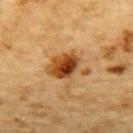biopsy_status: not biopsied; imaged during a skin examination
site: upper back
image:
  source: total-body photography crop
  field_of_view_mm: 15
patient:
  sex: male
  age_approx: 85+